A lesion tile, about 15 mm wide, cut from a 3D total-body photograph. Located on the back. A female subject, aged 18–22 — 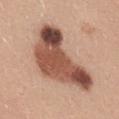Findings:
– lighting: white-light illumination
– TBP lesion metrics: a lesion color around L≈51 a*≈23 b*≈28 in CIELAB, a lesion–skin lightness drop of about 18, and a lesion-to-skin contrast of about 11.5 (normalized; higher = more distinct); a nevus-likeness score of about 0/100 and lesion-presence confidence of about 100/100
– histopathology: a dysplastic (Clark) nevus